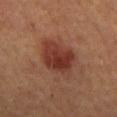notes: total-body-photography surveillance lesion; no biopsy | location: the abdomen | TBP lesion metrics: a mean CIELAB color near L≈33 a*≈22 b*≈25 and roughly 9 lightness units darker than nearby skin; a border-irregularity index near 3.5/10, a within-lesion color-variation index near 4.5/10, and radial color variation of about 1.5; an automated nevus-likeness rating near 100 out of 100 and lesion-presence confidence of about 100/100 | imaging modality: 15 mm crop, total-body photography | lighting: cross-polarized | subject: male, in their mid-60s.